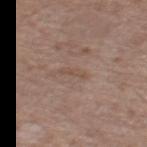The lesion was photographed on a routine skin check and not biopsied; there is no pathology result.
The subject is a female roughly 75 years of age.
A 15 mm crop from a total-body photograph taken for skin-cancer surveillance.
This is a white-light tile.
The lesion's longest dimension is about 3 mm.
The total-body-photography lesion software estimated a lesion color around L≈50 a*≈16 b*≈25 in CIELAB and a normalized lesion–skin contrast near 4.5. The analysis additionally found a border-irregularity index near 5/10, a color-variation rating of about 0/10, and radial color variation of about 0. The analysis additionally found a classifier nevus-likeness of about 0/100.
From the right thigh.